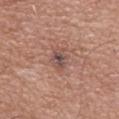– follow-up — imaged on a skin check; not biopsied
– site — the upper back
– image — 15 mm crop, total-body photography
– automated metrics — a border-irregularity rating of about 2/10, a color-variation rating of about 5/10, and a peripheral color-asymmetry measure near 1.5; a nevus-likeness score of about 0/100
– patient — male, aged 68 to 72
– size — ~3 mm (longest diameter)
– tile lighting — white-light